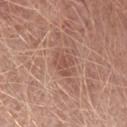The lesion was tiled from a total-body skin photograph and was not biopsied. The total-body-photography lesion software estimated an average lesion color of about L≈51 a*≈22 b*≈27 (CIELAB) and a lesion–skin lightness drop of about 8. The analysis additionally found a nevus-likeness score of about 5/100 and a detector confidence of about 100 out of 100 that the crop contains a lesion. Longest diameter approximately 3 mm. From the arm. A close-up tile cropped from a whole-body skin photograph, about 15 mm across. A male patient approximately 50 years of age. The tile uses white-light illumination.